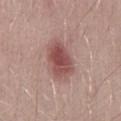Automated tile analysis of the lesion measured a symmetry-axis asymmetry near 0.2. It also reported border irregularity of about 2 on a 0–10 scale, a within-lesion color-variation index near 4/10, and a peripheral color-asymmetry measure near 1. The analysis additionally found a classifier nevus-likeness of about 85/100 and a lesion-detection confidence of about 100/100.
A roughly 15 mm field-of-view crop from a total-body skin photograph.
Measured at roughly 5 mm in maximum diameter.
This is a white-light tile.
A male patient, aged approximately 30.